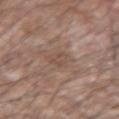The lesion was tiled from a total-body skin photograph and was not biopsied. About 3 mm across. A close-up tile cropped from a whole-body skin photograph, about 15 mm across. Located on the left forearm. The subject is a male aged 53 to 57.